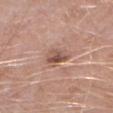A 15 mm crop from a total-body photograph taken for skin-cancer surveillance. A male subject roughly 50 years of age. The lesion is located on the left lower leg.